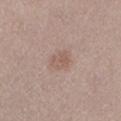Automated image analysis of the tile measured a footprint of about 5 mm², a shape eccentricity near 0.6, and a shape-asymmetry score of about 0.25 (0 = symmetric). The analysis additionally found a mean CIELAB color near L≈56 a*≈17 b*≈25, about 7 CIELAB-L* units darker than the surrounding skin, and a normalized border contrast of about 5. And it measured a classifier nevus-likeness of about 10/100 and a detector confidence of about 100 out of 100 that the crop contains a lesion. Captured under white-light illumination. The patient is a female approximately 45 years of age. Located on the left lower leg. The lesion's longest dimension is about 2.5 mm. A 15 mm close-up extracted from a 3D total-body photography capture.